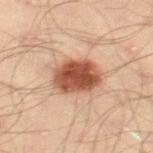biopsy status: imaged on a skin check; not biopsied
subject: male, about 45 years old
imaging modality: ~15 mm crop, total-body skin-cancer survey
illumination: cross-polarized illumination
TBP lesion metrics: a mean CIELAB color near L≈42 a*≈21 b*≈27 and a lesion-to-skin contrast of about 11.5 (normalized; higher = more distinct); an automated nevus-likeness rating near 100 out of 100
location: the right thigh
size: about 5 mm